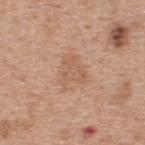subject = male, approximately 55 years of age
automated metrics = border irregularity of about 4.5 on a 0–10 scale and a peripheral color-asymmetry measure near 1; a lesion-detection confidence of about 100/100
imaging modality = total-body-photography crop, ~15 mm field of view
location = the back
illumination = white-light
lesion size = ~4 mm (longest diameter)